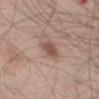The lesion was photographed on a routine skin check and not biopsied; there is no pathology result. The patient is a male aged around 60. Automated tile analysis of the lesion measured an area of roughly 5 mm² and a shape-asymmetry score of about 0.2 (0 = symmetric). Captured under white-light illumination. The lesion is on the leg. A 15 mm close-up tile from a total-body photography series done for melanoma screening.